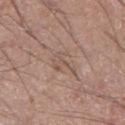The lesion was photographed on a routine skin check and not biopsied; there is no pathology result.
The lesion is located on the right lower leg.
A male subject approximately 60 years of age.
Measured at roughly 3.5 mm in maximum diameter.
A lesion tile, about 15 mm wide, cut from a 3D total-body photograph.